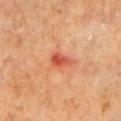Q: Was this lesion biopsied?
A: no biopsy performed (imaged during a skin exam)
Q: How large is the lesion?
A: about 3 mm
Q: What kind of image is this?
A: ~15 mm crop, total-body skin-cancer survey
Q: Lesion location?
A: the arm
Q: What are the patient's age and sex?
A: male, in their mid-60s
Q: Automated lesion metrics?
A: a lesion color around L≈53 a*≈35 b*≈37 in CIELAB and about 11 CIELAB-L* units darker than the surrounding skin; a color-variation rating of about 4/10 and radial color variation of about 1.5; a lesion-detection confidence of about 100/100